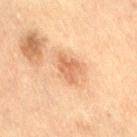Case summary:
• workup: imaged on a skin check; not biopsied
• lighting: cross-polarized illumination
• patient: female, aged 53–57
• image source: ~15 mm tile from a whole-body skin photo
• anatomic site: the right thigh